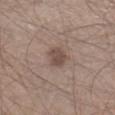Clinical summary: The lesion's longest dimension is about 3 mm. On the right lower leg. A male patient, about 45 years old. Automated tile analysis of the lesion measured a footprint of about 5 mm² and two-axis asymmetry of about 0.25. And it measured a mean CIELAB color near L≈47 a*≈15 b*≈22, a lesion–skin lightness drop of about 10, and a normalized lesion–skin contrast near 7.5. The software also gave a nevus-likeness score of about 45/100 and a detector confidence of about 100 out of 100 that the crop contains a lesion. Imaged with white-light lighting. Cropped from a whole-body photographic skin survey; the tile spans about 15 mm.